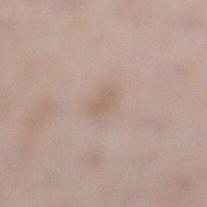This lesion was catalogued during total-body skin photography and was not selected for biopsy.
The patient is a female aged 38–42.
On the leg.
An algorithmic analysis of the crop reported a lesion color around L≈61 a*≈14 b*≈25 in CIELAB, about 6 CIELAB-L* units darker than the surrounding skin, and a normalized border contrast of about 4.5. The analysis additionally found an automated nevus-likeness rating near 5 out of 100 and a detector confidence of about 100 out of 100 that the crop contains a lesion.
The lesion's longest dimension is about 3 mm.
A 15 mm close-up extracted from a 3D total-body photography capture.